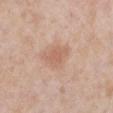Q: Is there a histopathology result?
A: total-body-photography surveillance lesion; no biopsy
Q: What is the lesion's diameter?
A: ~3.5 mm (longest diameter)
Q: What is the imaging modality?
A: 15 mm crop, total-body photography
Q: What did automated image analysis measure?
A: an average lesion color of about L≈62 a*≈21 b*≈30 (CIELAB), a lesion–skin lightness drop of about 8, and a normalized border contrast of about 5.5; a within-lesion color-variation index near 2/10; a nevus-likeness score of about 10/100
Q: Illumination type?
A: white-light illumination
Q: What is the anatomic site?
A: the right lower leg
Q: What are the patient's age and sex?
A: female, in their 60s